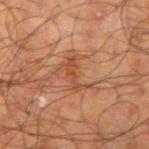Recorded during total-body skin imaging; not selected for excision or biopsy. A 15 mm crop from a total-body photograph taken for skin-cancer surveillance. A male patient aged around 70. Located on the right upper arm. About 4 mm across.